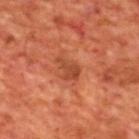{"biopsy_status": "not biopsied; imaged during a skin examination", "automated_metrics": {"border_irregularity_0_10": 5.0, "color_variation_0_10": 2.0, "peripheral_color_asymmetry": 0.5, "nevus_likeness_0_100": 45, "lesion_detection_confidence_0_100": 100}, "patient": {"sex": "male", "age_approx": 70}, "image": {"source": "total-body photography crop", "field_of_view_mm": 15}, "lesion_size": {"long_diameter_mm_approx": 3.0}, "lighting": "cross-polarized", "site": "upper back"}